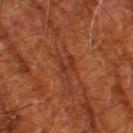Q: Is there a histopathology result?
A: catalogued during a skin exam; not biopsied